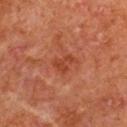Captured during whole-body skin photography for melanoma surveillance; the lesion was not biopsied. This image is a 15 mm lesion crop taken from a total-body photograph. The lesion is on the right upper arm. Measured at roughly 3 mm in maximum diameter. A male subject roughly 65 years of age. The tile uses cross-polarized illumination.